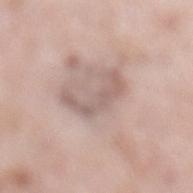Part of a total-body skin-imaging series; this lesion was reviewed on a skin check and was not flagged for biopsy.
A female subject, aged 68–72.
This is a white-light tile.
Measured at roughly 4.5 mm in maximum diameter.
From the left lower leg.
A lesion tile, about 15 mm wide, cut from a 3D total-body photograph.
An algorithmic analysis of the crop reported an area of roughly 6.5 mm², a shape eccentricity near 0.85, and a shape-asymmetry score of about 0.75 (0 = symmetric). The analysis additionally found an average lesion color of about L≈60 a*≈16 b*≈22 (CIELAB), about 9 CIELAB-L* units darker than the surrounding skin, and a lesion-to-skin contrast of about 6 (normalized; higher = more distinct). It also reported peripheral color asymmetry of about 0. It also reported a classifier nevus-likeness of about 0/100 and lesion-presence confidence of about 55/100.